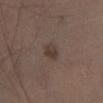Assessment:
The lesion was photographed on a routine skin check and not biopsied; there is no pathology result.
Image and clinical context:
Automated image analysis of the tile measured an area of roughly 4 mm², an eccentricity of roughly 0.55, and two-axis asymmetry of about 0.2. The software also gave roughly 8 lightness units darker than nearby skin and a normalized lesion–skin contrast near 7. And it measured a border-irregularity index near 2/10, a within-lesion color-variation index near 2.5/10, and a peripheral color-asymmetry measure near 1. And it measured an automated nevus-likeness rating near 40 out of 100. Longest diameter approximately 2.5 mm. A close-up tile cropped from a whole-body skin photograph, about 15 mm across. The subject is a male roughly 75 years of age. Located on the leg.